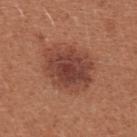<lesion>
  <automated_metrics>
    <cielab_L>43</cielab_L>
    <cielab_a>24</cielab_a>
    <cielab_b>28</cielab_b>
    <vs_skin_darker_L>11.0</vs_skin_darker_L>
    <vs_skin_contrast_norm>8.5</vs_skin_contrast_norm>
    <border_irregularity_0_10>2.0</border_irregularity_0_10>
    <color_variation_0_10>4.5</color_variation_0_10>
    <peripheral_color_asymmetry>1.0</peripheral_color_asymmetry>
  </automated_metrics>
  <site>chest</site>
  <lighting>white-light</lighting>
  <patient>
    <sex>female</sex>
    <age_approx>35</age_approx>
  </patient>
  <image>
    <source>total-body photography crop</source>
    <field_of_view_mm>15</field_of_view_mm>
  </image>
</lesion>